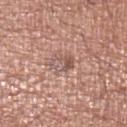notes: catalogued during a skin exam; not biopsied
patient: male, roughly 70 years of age
location: the left lower leg
automated lesion analysis: a mean CIELAB color near L≈55 a*≈20 b*≈23, roughly 9 lightness units darker than nearby skin, and a normalized lesion–skin contrast near 6.5; a border-irregularity index near 2.5/10, a within-lesion color-variation index near 8.5/10, and peripheral color asymmetry of about 3
lesion size: ≈3 mm
imaging modality: ~15 mm tile from a whole-body skin photo